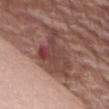  biopsy_status: not biopsied; imaged during a skin examination
  image:
    source: total-body photography crop
    field_of_view_mm: 15
  lighting: white-light
  automated_metrics:
    cielab_L: 43
    cielab_a: 21
    cielab_b: 23
    vs_skin_darker_L: 11.0
    vs_skin_contrast_norm: 8.5
    border_irregularity_0_10: 7.0
    color_variation_0_10: 7.5
    peripheral_color_asymmetry: 2.5
    nevus_likeness_0_100: 0
    lesion_detection_confidence_0_100: 80
  lesion_size:
    long_diameter_mm_approx: 7.5
  site: front of the torso
  patient:
    sex: female
    age_approx: 75